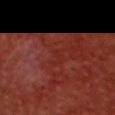{
  "biopsy_status": "not biopsied; imaged during a skin examination",
  "image": {
    "source": "total-body photography crop",
    "field_of_view_mm": 15
  },
  "lighting": "cross-polarized",
  "site": "head or neck",
  "patient": {
    "sex": "male",
    "age_approx": 60
  },
  "lesion_size": {
    "long_diameter_mm_approx": 2.0
  }
}